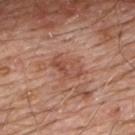Q: Is there a histopathology result?
A: total-body-photography surveillance lesion; no biopsy
Q: Lesion size?
A: ≈3.5 mm
Q: What is the imaging modality?
A: ~15 mm tile from a whole-body skin photo
Q: Where on the body is the lesion?
A: the upper back
Q: Patient demographics?
A: male, aged 78 to 82